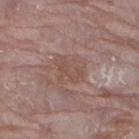Impression:
The lesion was tiled from a total-body skin photograph and was not biopsied.
Background:
On the left lower leg. A female patient, aged 63 to 67. A 15 mm close-up extracted from a 3D total-body photography capture. Imaged with white-light lighting. Measured at roughly 3.5 mm in maximum diameter.